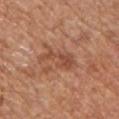Clinical impression: This lesion was catalogued during total-body skin photography and was not selected for biopsy. Context: The lesion's longest dimension is about 5 mm. The lesion-visualizer software estimated a mean CIELAB color near L≈49 a*≈24 b*≈32, about 9 CIELAB-L* units darker than the surrounding skin, and a normalized border contrast of about 6.5. The software also gave a nevus-likeness score of about 0/100 and lesion-presence confidence of about 100/100. The tile uses white-light illumination. A region of skin cropped from a whole-body photographic capture, roughly 15 mm wide. From the chest. The subject is a male roughly 65 years of age.